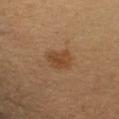Part of a total-body skin-imaging series; this lesion was reviewed on a skin check and was not flagged for biopsy. A lesion tile, about 15 mm wide, cut from a 3D total-body photograph. Captured under cross-polarized illumination. Longest diameter approximately 3 mm. An algorithmic analysis of the crop reported a mean CIELAB color near L≈38 a*≈17 b*≈31, about 7 CIELAB-L* units darker than the surrounding skin, and a normalized border contrast of about 7. It also reported a border-irregularity index near 2.5/10, a within-lesion color-variation index near 2/10, and peripheral color asymmetry of about 0.5. On the left upper arm. The patient is a male in their mid- to late 50s.